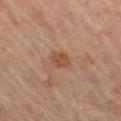- notes — imaged on a skin check; not biopsied
- site — the leg
- acquisition — ~15 mm tile from a whole-body skin photo
- diameter — about 2.5 mm
- automated metrics — a mean CIELAB color near L≈46 a*≈20 b*≈31, roughly 8 lightness units darker than nearby skin, and a normalized lesion–skin contrast near 7; a border-irregularity rating of about 2/10, a color-variation rating of about 1.5/10, and peripheral color asymmetry of about 0.5
- subject — male, in their mid- to late 60s
- tile lighting — cross-polarized illumination